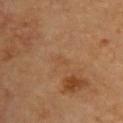follow-up — catalogued during a skin exam; not biopsied
tile lighting — cross-polarized
anatomic site — the back
patient — female, aged approximately 70
image-analysis metrics — an outline eccentricity of about 0.85 (0 = round, 1 = elongated) and a symmetry-axis asymmetry near 0.45; a classifier nevus-likeness of about 0/100 and lesion-presence confidence of about 100/100
lesion diameter — about 2 mm
acquisition — total-body-photography crop, ~15 mm field of view